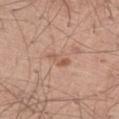The lesion was tiled from a total-body skin photograph and was not biopsied. Imaged with white-light lighting. A close-up tile cropped from a whole-body skin photograph, about 15 mm across. A male patient, about 40 years old. From the left thigh. About 3 mm across.